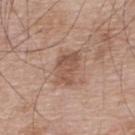Captured during whole-body skin photography for melanoma surveillance; the lesion was not biopsied. About 4 mm across. The tile uses white-light illumination. Cropped from a whole-body photographic skin survey; the tile spans about 15 mm. The patient is a male aged approximately 65. From the upper back.